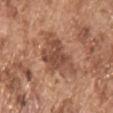Q: Was this lesion biopsied?
A: imaged on a skin check; not biopsied
Q: What is the anatomic site?
A: the chest
Q: How was the tile lit?
A: white-light illumination
Q: What is the lesion's diameter?
A: about 6 mm
Q: Who is the patient?
A: male, approximately 75 years of age
Q: What is the imaging modality?
A: ~15 mm crop, total-body skin-cancer survey
Q: What did automated image analysis measure?
A: a mean CIELAB color near L≈48 a*≈22 b*≈30, a lesion–skin lightness drop of about 10, and a lesion-to-skin contrast of about 7.5 (normalized; higher = more distinct); a nevus-likeness score of about 0/100 and a lesion-detection confidence of about 100/100